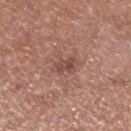– notes — no biopsy performed (imaged during a skin exam)
– subject — male, aged around 70
– lighting — white-light illumination
– site — the leg
– automated lesion analysis — an area of roughly 4 mm², a shape eccentricity near 0.75, and a shape-asymmetry score of about 0.25 (0 = symmetric); internal color variation of about 4 on a 0–10 scale and a peripheral color-asymmetry measure near 1.5
– image — total-body-photography crop, ~15 mm field of view